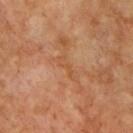biopsy status: no biopsy performed (imaged during a skin exam)
patient: male, roughly 65 years of age
image source: ~15 mm tile from a whole-body skin photo
automated metrics: a lesion area of about 3 mm², an outline eccentricity of about 0.95 (0 = round, 1 = elongated), and a shape-asymmetry score of about 0.5 (0 = symmetric); an average lesion color of about L≈51 a*≈23 b*≈37 (CIELAB) and roughly 6 lightness units darker than nearby skin; border irregularity of about 6.5 on a 0–10 scale, a color-variation rating of about 0/10, and a peripheral color-asymmetry measure near 0; a lesion-detection confidence of about 85/100
illumination: cross-polarized illumination
size: ≈3.5 mm